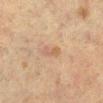{"biopsy_status": "not biopsied; imaged during a skin examination", "automated_metrics": {"area_mm2_approx": 3.0, "shape_asymmetry": 0.35, "cielab_L": 48, "cielab_a": 16, "cielab_b": 27, "vs_skin_darker_L": 6.0, "vs_skin_contrast_norm": 5.0, "border_irregularity_0_10": 3.5, "color_variation_0_10": 0.0, "nevus_likeness_0_100": 0, "lesion_detection_confidence_0_100": 100}, "lighting": "cross-polarized", "site": "left lower leg", "lesion_size": {"long_diameter_mm_approx": 2.5}, "image": {"source": "total-body photography crop", "field_of_view_mm": 15}, "patient": {"sex": "female", "age_approx": 55}}